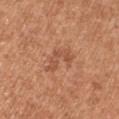Clinical impression: Captured during whole-body skin photography for melanoma surveillance; the lesion was not biopsied. Clinical summary: A roughly 15 mm field-of-view crop from a total-body skin photograph. The lesion is on the right upper arm. A male subject, aged around 55.